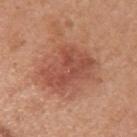Assessment:
Imaged during a routine full-body skin examination; the lesion was not biopsied and no histopathology is available.
Context:
A female patient, aged around 50. Approximately 6 mm at its widest. Captured under white-light illumination. The lesion is located on the left upper arm. A 15 mm crop from a total-body photograph taken for skin-cancer surveillance.